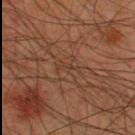workup = imaged on a skin check; not biopsied | imaging modality = total-body-photography crop, ~15 mm field of view | patient = male, aged around 45 | site = the back.